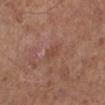| field | value |
|---|---|
| workup | imaged on a skin check; not biopsied |
| subject | male, aged around 60 |
| automated lesion analysis | a lesion area of about 3.5 mm² and an outline eccentricity of about 0.85 (0 = round, 1 = elongated); a border-irregularity rating of about 2.5/10, a within-lesion color-variation index near 1/10, and a peripheral color-asymmetry measure near 0.5; a nevus-likeness score of about 0/100 and a detector confidence of about 100 out of 100 that the crop contains a lesion |
| body site | the left lower leg |
| illumination | white-light illumination |
| image source | total-body-photography crop, ~15 mm field of view |